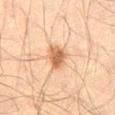follow-up: no biopsy performed (imaged during a skin exam) | illumination: cross-polarized illumination | patient: male, roughly 60 years of age | diameter: about 3 mm | image-analysis metrics: a classifier nevus-likeness of about 90/100 | image: ~15 mm crop, total-body skin-cancer survey | anatomic site: the left thigh.